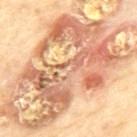follow-up: total-body-photography surveillance lesion; no biopsy | anatomic site: the back | automated metrics: a lesion color around L≈70 a*≈20 b*≈36 in CIELAB, about 14 CIELAB-L* units darker than the surrounding skin, and a lesion-to-skin contrast of about 8 (normalized; higher = more distinct); a border-irregularity index near 5/10 and a color-variation rating of about 10/10; a nevus-likeness score of about 0/100 and a lesion-detection confidence of about 5/100 | acquisition: ~15 mm crop, total-body skin-cancer survey | lesion diameter: ≈19.5 mm | patient: male, about 70 years old.